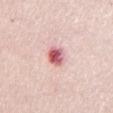<case>
  <biopsy_status>not biopsied; imaged during a skin examination</biopsy_status>
  <lesion_size>
    <long_diameter_mm_approx>3.0</long_diameter_mm_approx>
  </lesion_size>
  <patient>
    <sex>female</sex>
    <age_approx>45</age_approx>
  </patient>
  <image>
    <source>total-body photography crop</source>
    <field_of_view_mm>15</field_of_view_mm>
  </image>
  <site>chest</site>
  <automated_metrics>
    <vs_skin_darker_L>18.0</vs_skin_darker_L>
    <color_variation_0_10>8.0</color_variation_0_10>
    <peripheral_color_asymmetry>2.5</peripheral_color_asymmetry>
  </automated_metrics>
</case>